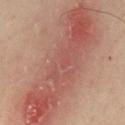Impression:
Part of a total-body skin-imaging series; this lesion was reviewed on a skin check and was not flagged for biopsy.
Context:
A region of skin cropped from a whole-body photographic capture, roughly 15 mm wide. A male patient, roughly 55 years of age. On the mid back.